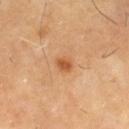<tbp_lesion>
<biopsy_status>not biopsied; imaged during a skin examination</biopsy_status>
<site>left thigh</site>
<image>
  <source>total-body photography crop</source>
  <field_of_view_mm>15</field_of_view_mm>
</image>
<lesion_size>
  <long_diameter_mm_approx>2.0</long_diameter_mm_approx>
</lesion_size>
<lighting>cross-polarized</lighting>
<patient>
  <sex>male</sex>
  <age_approx>60</age_approx>
</patient>
</tbp_lesion>